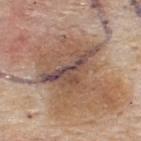Notes:
* notes: no biopsy performed (imaged during a skin exam)
* location: the upper back
* image source: total-body-photography crop, ~15 mm field of view
* automated metrics: an eccentricity of roughly 0.9; a classifier nevus-likeness of about 0/100
* tile lighting: white-light illumination
* subject: male, aged 53 to 57
* diameter: ≈7 mm